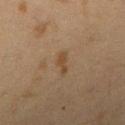biopsy status: total-body-photography surveillance lesion; no biopsy
automated lesion analysis: a shape eccentricity near 0.9 and a shape-asymmetry score of about 0.35 (0 = symmetric); border irregularity of about 4.5 on a 0–10 scale, a color-variation rating of about 0.5/10, and peripheral color asymmetry of about 0; a nevus-likeness score of about 5/100 and a detector confidence of about 100 out of 100 that the crop contains a lesion
size: about 2.5 mm
location: the arm
subject: female, aged 38–42
image: ~15 mm crop, total-body skin-cancer survey